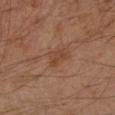{"lighting": "cross-polarized", "patient": {"sex": "male", "age_approx": 45}, "site": "arm", "image": {"source": "total-body photography crop", "field_of_view_mm": 15}, "lesion_size": {"long_diameter_mm_approx": 3.0}, "automated_metrics": {"area_mm2_approx": 4.0, "eccentricity": 0.75, "shape_asymmetry": 0.4, "border_irregularity_0_10": 4.0, "color_variation_0_10": 1.0, "peripheral_color_asymmetry": 0.5, "nevus_likeness_0_100": 0, "lesion_detection_confidence_0_100": 100}}